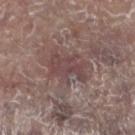Assessment:
The lesion was tiled from a total-body skin photograph and was not biopsied.
Clinical summary:
This image is a 15 mm lesion crop taken from a total-body photograph. About 5.5 mm across. A male subject in their 80s. This is a white-light tile. The lesion-visualizer software estimated a footprint of about 11 mm², an outline eccentricity of about 0.8 (0 = round, 1 = elongated), and two-axis asymmetry of about 0.4. It also reported a border-irregularity rating of about 6.5/10 and a within-lesion color-variation index near 2/10. The software also gave an automated nevus-likeness rating near 0 out of 100. The lesion is on the leg.